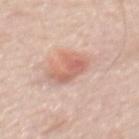workup = catalogued during a skin exam; not biopsied.